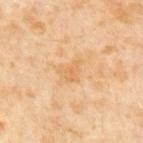Assessment:
The lesion was tiled from a total-body skin photograph and was not biopsied.
Clinical summary:
An algorithmic analysis of the crop reported an area of roughly 4.5 mm² and a shape-asymmetry score of about 0.55 (0 = symmetric). And it measured a lesion-detection confidence of about 100/100. A lesion tile, about 15 mm wide, cut from a 3D total-body photograph. The tile uses cross-polarized illumination. The recorded lesion diameter is about 3 mm. The subject is a male aged approximately 65.